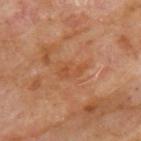{
  "biopsy_status": "not biopsied; imaged during a skin examination",
  "image": {
    "source": "total-body photography crop",
    "field_of_view_mm": 15
  },
  "lesion_size": {
    "long_diameter_mm_approx": 3.5
  },
  "patient": {
    "sex": "male",
    "age_approx": 70
  },
  "site": "upper back",
  "automated_metrics": {
    "area_mm2_approx": 5.0,
    "shape_asymmetry": 0.3,
    "vs_skin_darker_L": 5.0,
    "vs_skin_contrast_norm": 5.0,
    "border_irregularity_0_10": 3.0,
    "color_variation_0_10": 2.5,
    "peripheral_color_asymmetry": 1.0
  }
}